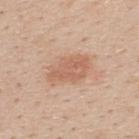Part of a total-body skin-imaging series; this lesion was reviewed on a skin check and was not flagged for biopsy. Automated image analysis of the tile measured a border-irregularity index near 2.5/10, internal color variation of about 2.5 on a 0–10 scale, and a peripheral color-asymmetry measure near 0.5. On the back. Cropped from a whole-body photographic skin survey; the tile spans about 15 mm. About 5 mm across. The tile uses white-light illumination. The patient is a male approximately 30 years of age.